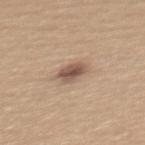Imaged during a routine full-body skin examination; the lesion was not biopsied and no histopathology is available. Automated tile analysis of the lesion measured an area of roughly 6.5 mm² and a shape eccentricity near 0.65. Imaged with white-light lighting. A female patient aged 28 to 32. The lesion is on the upper back. Cropped from a whole-body photographic skin survey; the tile spans about 15 mm. Approximately 3.5 mm at its widest.